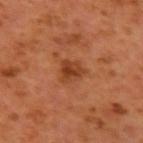  biopsy_status: not biopsied; imaged during a skin examination
  lesion_size:
    long_diameter_mm_approx: 3.0
  patient:
    sex: male
    age_approx: 60
  site: left upper arm
  lighting: cross-polarized
  image:
    source: total-body photography crop
    field_of_view_mm: 15